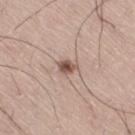Impression:
The lesion was photographed on a routine skin check and not biopsied; there is no pathology result.
Acquisition and patient details:
Imaged with white-light lighting. On the right thigh. The total-body-photography lesion software estimated a footprint of about 4 mm², a shape eccentricity near 0.65, and a symmetry-axis asymmetry near 0.25. And it measured a lesion color around L≈54 a*≈17 b*≈24 in CIELAB, roughly 13 lightness units darker than nearby skin, and a normalized border contrast of about 8.5. And it measured a nevus-likeness score of about 95/100 and lesion-presence confidence of about 100/100. A male subject aged 58 to 62. A region of skin cropped from a whole-body photographic capture, roughly 15 mm wide. The lesion's longest dimension is about 2.5 mm.